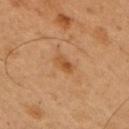  biopsy_status: not biopsied; imaged during a skin examination
  site: right upper arm
  image:
    source: total-body photography crop
    field_of_view_mm: 15
  lesion_size:
    long_diameter_mm_approx: 3.0
  patient:
    sex: male
    age_approx: 50